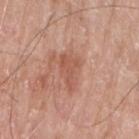No biopsy was performed on this lesion — it was imaged during a full skin examination and was not determined to be concerning. Approximately 4 mm at its widest. A male subject, aged around 85 or older. Imaged with white-light lighting. The lesion is located on the right upper arm. The lesion-visualizer software estimated a border-irregularity rating of about 6/10 and peripheral color asymmetry of about 0.5. Cropped from a total-body skin-imaging series; the visible field is about 15 mm.